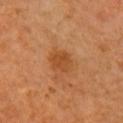Assessment:
Part of a total-body skin-imaging series; this lesion was reviewed on a skin check and was not flagged for biopsy.
Clinical summary:
A male patient aged 58–62. Longest diameter approximately 3 mm. This image is a 15 mm lesion crop taken from a total-body photograph. The lesion is on the right upper arm. Captured under cross-polarized illumination.